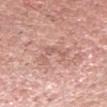follow-up: imaged on a skin check; not biopsied
anatomic site: the head or neck
acquisition: total-body-photography crop, ~15 mm field of view
patient: male, aged 53 to 57